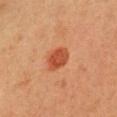Notes:
– follow-up — imaged on a skin check; not biopsied
– subject — female, aged 38–42
– size — ~3 mm (longest diameter)
– image source — ~15 mm crop, total-body skin-cancer survey
– tile lighting — cross-polarized illumination
– body site — the head or neck